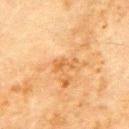Q: Was this lesion biopsied?
A: no biopsy performed (imaged during a skin exam)
Q: Where on the body is the lesion?
A: the chest
Q: What kind of image is this?
A: total-body-photography crop, ~15 mm field of view
Q: How large is the lesion?
A: ≈3.5 mm
Q: Automated lesion metrics?
A: peripheral color asymmetry of about 0; a nevus-likeness score of about 0/100 and a detector confidence of about 100 out of 100 that the crop contains a lesion
Q: What lighting was used for the tile?
A: cross-polarized illumination
Q: What are the patient's age and sex?
A: male, aged around 70